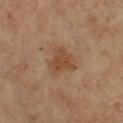Notes:
– acquisition: 15 mm crop, total-body photography
– site: the right lower leg
– automated lesion analysis: a footprint of about 9 mm², an eccentricity of roughly 0.5, and a symmetry-axis asymmetry near 0.4; a mean CIELAB color near L≈44 a*≈18 b*≈31; border irregularity of about 4 on a 0–10 scale, a within-lesion color-variation index near 2.5/10, and radial color variation of about 1; a nevus-likeness score of about 70/100 and lesion-presence confidence of about 100/100
– subject: female, aged approximately 70
– lesion size: ~3.5 mm (longest diameter)